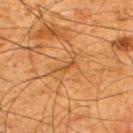Part of a total-body skin-imaging series; this lesion was reviewed on a skin check and was not flagged for biopsy.
On the upper back.
The patient is a male in their mid- to late 60s.
Cropped from a whole-body photographic skin survey; the tile spans about 15 mm.
The total-body-photography lesion software estimated a footprint of about 2 mm², a shape eccentricity near 0.9, and a shape-asymmetry score of about 0.4 (0 = symmetric). And it measured an average lesion color of about L≈43 a*≈22 b*≈37 (CIELAB), a lesion–skin lightness drop of about 7, and a lesion-to-skin contrast of about 5.5 (normalized; higher = more distinct). It also reported border irregularity of about 4.5 on a 0–10 scale, a within-lesion color-variation index near 0/10, and peripheral color asymmetry of about 0.
This is a cross-polarized tile.
Longest diameter approximately 2.5 mm.